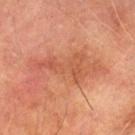The lesion is located on the left thigh. A male subject, roughly 75 years of age. A lesion tile, about 15 mm wide, cut from a 3D total-body photograph.